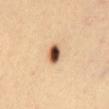notes: total-body-photography surveillance lesion; no biopsy | location: the chest | imaging modality: ~15 mm tile from a whole-body skin photo | tile lighting: cross-polarized | TBP lesion metrics: a lesion area of about 4.5 mm², an outline eccentricity of about 0.65 (0 = round, 1 = elongated), and two-axis asymmetry of about 0.2; a mean CIELAB color near L≈52 a*≈20 b*≈33 and a normalized lesion–skin contrast near 14; a border-irregularity rating of about 1.5/10, a color-variation rating of about 10/10, and a peripheral color-asymmetry measure near 3 | patient: female, roughly 35 years of age.